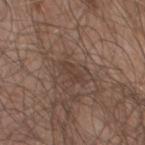The lesion was tiled from a total-body skin photograph and was not biopsied.
Approximately 2.5 mm at its widest.
A close-up tile cropped from a whole-body skin photograph, about 15 mm across.
A male patient, aged around 65.
Automated image analysis of the tile measured an area of roughly 3 mm², an outline eccentricity of about 0.85 (0 = round, 1 = elongated), and a shape-asymmetry score of about 0.25 (0 = symmetric). The analysis additionally found a border-irregularity rating of about 3/10 and radial color variation of about 0.5. And it measured a nevus-likeness score of about 0/100.
This is a white-light tile.
The lesion is on the chest.